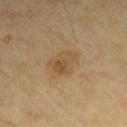| feature | finding |
|---|---|
| workup | catalogued during a skin exam; not biopsied |